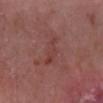Q: What kind of image is this?
A: ~15 mm tile from a whole-body skin photo
Q: Who is the patient?
A: male, in their mid- to late 60s
Q: Lesion location?
A: the right lower leg
Q: What is the lesion's diameter?
A: ≈4 mm
Q: Illumination type?
A: white-light illumination